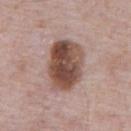Recorded during total-body skin imaging; not selected for excision or biopsy. The patient is a male aged approximately 70. On the abdomen. About 6 mm across. Cropped from a whole-body photographic skin survey; the tile spans about 15 mm. Imaged with white-light lighting. Automated image analysis of the tile measured a footprint of about 22 mm² and an eccentricity of roughly 0.75. It also reported a lesion color around L≈48 a*≈19 b*≈24 in CIELAB, a lesion–skin lightness drop of about 16, and a normalized lesion–skin contrast near 11.5. It also reported a border-irregularity rating of about 1.5/10, a within-lesion color-variation index near 9/10, and a peripheral color-asymmetry measure near 3.5.